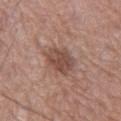{
  "biopsy_status": "not biopsied; imaged during a skin examination",
  "automated_metrics": {
    "nevus_likeness_0_100": 30
  },
  "site": "left lower leg",
  "lighting": "white-light",
  "lesion_size": {
    "long_diameter_mm_approx": 4.0
  },
  "image": {
    "source": "total-body photography crop",
    "field_of_view_mm": 15
  },
  "patient": {
    "sex": "male",
    "age_approx": 60
  }
}